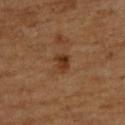A lesion tile, about 15 mm wide, cut from a 3D total-body photograph.
Located on the back.
The patient is a male aged 58 to 62.